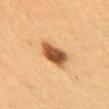The lesion was tiled from a total-body skin photograph and was not biopsied. A 15 mm crop from a total-body photograph taken for skin-cancer surveillance. From the arm. Captured under cross-polarized illumination. A female subject, approximately 40 years of age.